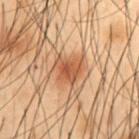– notes: no biopsy performed (imaged during a skin exam)
– lesion size: about 3.5 mm
– acquisition: ~15 mm crop, total-body skin-cancer survey
– patient: male, aged 53–57
– lighting: cross-polarized illumination
– anatomic site: the abdomen
– TBP lesion metrics: a border-irregularity index near 3/10, a color-variation rating of about 4/10, and radial color variation of about 1; a detector confidence of about 100 out of 100 that the crop contains a lesion